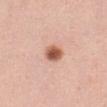follow-up=no biopsy performed (imaged during a skin exam) | patient=female, about 45 years old | lesion diameter=≈2.5 mm | anatomic site=the abdomen | acquisition=total-body-photography crop, ~15 mm field of view.